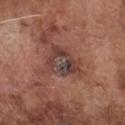Assessment: No biopsy was performed on this lesion — it was imaged during a full skin examination and was not determined to be concerning. Image and clinical context: Located on the chest. A 15 mm crop from a total-body photograph taken for skin-cancer surveillance. Measured at roughly 5 mm in maximum diameter. The tile uses white-light illumination. The subject is a male about 75 years old.